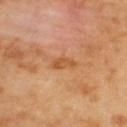Background:
The subject is a female aged approximately 60. On the back. Cropped from a whole-body photographic skin survey; the tile spans about 15 mm. This is a cross-polarized tile.